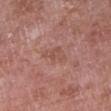The lesion was tiled from a total-body skin photograph and was not biopsied. The subject is a female in their 70s. Located on the right upper arm. This image is a 15 mm lesion crop taken from a total-body photograph.